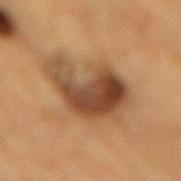This lesion was catalogued during total-body skin photography and was not selected for biopsy.
The recorded lesion diameter is about 7.5 mm.
From the mid back.
Automated tile analysis of the lesion measured a mean CIELAB color near L≈40 a*≈17 b*≈30 and a lesion-to-skin contrast of about 10 (normalized; higher = more distinct). The analysis additionally found a nevus-likeness score of about 50/100 and a lesion-detection confidence of about 100/100.
A 15 mm close-up extracted from a 3D total-body photography capture.
The tile uses cross-polarized illumination.
The patient is a male in their mid-80s or older.